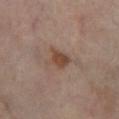This lesion was catalogued during total-body skin photography and was not selected for biopsy. Approximately 3 mm at its widest. The subject is a male approximately 60 years of age. Cropped from a total-body skin-imaging series; the visible field is about 15 mm. From the left lower leg.